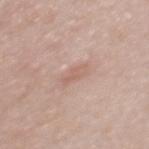Imaged during a routine full-body skin examination; the lesion was not biopsied and no histopathology is available.
Captured under white-light illumination.
Automated image analysis of the tile measured a symmetry-axis asymmetry near 0.3. It also reported a lesion color around L≈61 a*≈20 b*≈26 in CIELAB and about 7 CIELAB-L* units darker than the surrounding skin.
Located on the upper back.
The lesion's longest dimension is about 3 mm.
The patient is a female in their 40s.
A region of skin cropped from a whole-body photographic capture, roughly 15 mm wide.